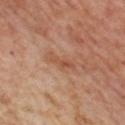acquisition — total-body-photography crop, ~15 mm field of view | anatomic site — the left thigh | patient — female, about 55 years old.